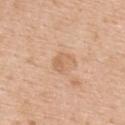Impression: This lesion was catalogued during total-body skin photography and was not selected for biopsy. Context: About 2.5 mm across. The patient is a female aged 63 to 67. A 15 mm close-up extracted from a 3D total-body photography capture. An algorithmic analysis of the crop reported an area of roughly 4.5 mm², an eccentricity of roughly 0.35, and two-axis asymmetry of about 0.35. And it measured an average lesion color of about L≈64 a*≈20 b*≈35 (CIELAB) and a lesion–skin lightness drop of about 7. The analysis additionally found a nevus-likeness score of about 0/100 and lesion-presence confidence of about 100/100. On the back.